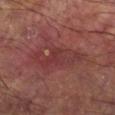biopsy_status: not biopsied; imaged during a skin examination
image:
  source: total-body photography crop
  field_of_view_mm: 15
lighting: cross-polarized
lesion_size:
  long_diameter_mm_approx: 6.5
site: leg
patient:
  sex: male
  age_approx: 60